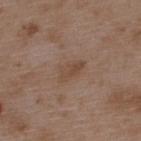Clinical impression: Part of a total-body skin-imaging series; this lesion was reviewed on a skin check and was not flagged for biopsy. Clinical summary: The recorded lesion diameter is about 3.5 mm. A male patient aged 48–52. Automated tile analysis of the lesion measured a footprint of about 6 mm², an outline eccentricity of about 0.8 (0 = round, 1 = elongated), and two-axis asymmetry of about 0.25. And it measured an average lesion color of about L≈47 a*≈16 b*≈27 (CIELAB), about 6 CIELAB-L* units darker than the surrounding skin, and a lesion-to-skin contrast of about 5.5 (normalized; higher = more distinct). From the back. A close-up tile cropped from a whole-body skin photograph, about 15 mm across. The tile uses white-light illumination.